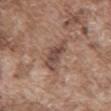<case>
<automated_metrics>
  <area_mm2_approx>8.0</area_mm2_approx>
  <eccentricity>0.85</eccentricity>
  <shape_asymmetry>0.4</shape_asymmetry>
  <cielab_L>47</cielab_L>
  <cielab_a>18</cielab_a>
  <cielab_b>24</cielab_b>
  <vs_skin_darker_L>10.0</vs_skin_darker_L>
  <vs_skin_contrast_norm>8.0</vs_skin_contrast_norm>
  <color_variation_0_10>3.5</color_variation_0_10>
  <peripheral_color_asymmetry>1.0</peripheral_color_asymmetry>
  <nevus_likeness_0_100>0</nevus_likeness_0_100>
  <lesion_detection_confidence_0_100>85</lesion_detection_confidence_0_100>
</automated_metrics>
<patient>
  <sex>male</sex>
  <age_approx>75</age_approx>
</patient>
<site>back</site>
<image>
  <source>total-body photography crop</source>
  <field_of_view_mm>15</field_of_view_mm>
</image>
<lighting>white-light</lighting>
</case>